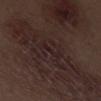Q: Was this lesion biopsied?
A: total-body-photography surveillance lesion; no biopsy
Q: Lesion location?
A: the left thigh
Q: How was the tile lit?
A: white-light illumination
Q: How was this image acquired?
A: 15 mm crop, total-body photography
Q: Who is the patient?
A: male, about 70 years old